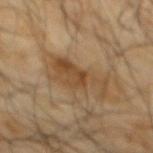workup=total-body-photography surveillance lesion; no biopsy
subject=male, aged around 65
imaging modality=~15 mm tile from a whole-body skin photo
anatomic site=the mid back